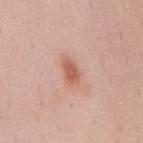This lesion was catalogued during total-body skin photography and was not selected for biopsy.
A close-up tile cropped from a whole-body skin photograph, about 15 mm across.
A female subject, aged 38–42.
On the mid back.
An algorithmic analysis of the crop reported a border-irregularity index near 2.5/10, a within-lesion color-variation index near 2/10, and a peripheral color-asymmetry measure near 0.5.
Captured under white-light illumination.
Approximately 3.5 mm at its widest.